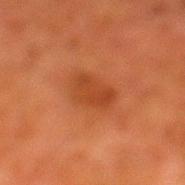Context: The lesion's longest dimension is about 4 mm. Automated tile analysis of the lesion measured an average lesion color of about L≈35 a*≈26 b*≈34 (CIELAB), a lesion–skin lightness drop of about 6, and a normalized border contrast of about 6. The analysis additionally found a classifier nevus-likeness of about 0/100 and a detector confidence of about 100 out of 100 that the crop contains a lesion. A male subject, aged 78 to 82. Imaged with cross-polarized lighting. A 15 mm close-up extracted from a 3D total-body photography capture. On the left lower leg.